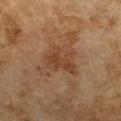workup = no biopsy performed (imaged during a skin exam)
automated lesion analysis = an area of roughly 10 mm² and two-axis asymmetry of about 0.35; a border-irregularity index near 4/10, a color-variation rating of about 2.5/10, and peripheral color asymmetry of about 1; a nevus-likeness score of about 0/100
lesion diameter = ≈4.5 mm
body site = the left forearm
imaging modality = 15 mm crop, total-body photography
illumination = cross-polarized
subject = female, aged approximately 60